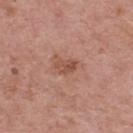follow-up = imaged on a skin check; not biopsied | body site = the upper back | patient = male, roughly 65 years of age | acquisition = ~15 mm crop, total-body skin-cancer survey.